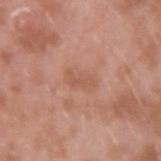Imaged during a routine full-body skin examination; the lesion was not biopsied and no histopathology is available.
A male subject approximately 40 years of age.
A 15 mm crop from a total-body photograph taken for skin-cancer surveillance.
Located on the left forearm.